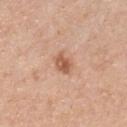Assessment: Captured during whole-body skin photography for melanoma surveillance; the lesion was not biopsied. Acquisition and patient details: The recorded lesion diameter is about 2.5 mm. A 15 mm crop from a total-body photograph taken for skin-cancer surveillance. On the chest. The lesion-visualizer software estimated a mean CIELAB color near L≈57 a*≈23 b*≈33 and a lesion–skin lightness drop of about 12. It also reported border irregularity of about 2 on a 0–10 scale, internal color variation of about 3.5 on a 0–10 scale, and radial color variation of about 1.5. The analysis additionally found a nevus-likeness score of about 85/100. A male patient about 40 years old.